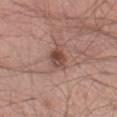{"biopsy_status": "not biopsied; imaged during a skin examination", "image": {"source": "total-body photography crop", "field_of_view_mm": 15}, "patient": {"sex": "male", "age_approx": 40}, "site": "right thigh"}